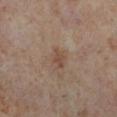biopsy status: total-body-photography surveillance lesion; no biopsy
automated lesion analysis: an area of roughly 3.5 mm², an outline eccentricity of about 0.8 (0 = round, 1 = elongated), and a shape-asymmetry score of about 0.2 (0 = symmetric); about 7 CIELAB-L* units darker than the surrounding skin and a normalized lesion–skin contrast near 6; a border-irregularity rating of about 2.5/10, a color-variation rating of about 2.5/10, and peripheral color asymmetry of about 1
tile lighting: cross-polarized
patient: female, in their 50s
image source: ~15 mm tile from a whole-body skin photo
lesion diameter: ~2.5 mm (longest diameter)
body site: the right lower leg